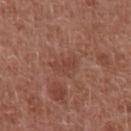The lesion was photographed on a routine skin check and not biopsied; there is no pathology result. Automated image analysis of the tile measured a lesion area of about 4 mm², an eccentricity of roughly 0.85, and a shape-asymmetry score of about 0.35 (0 = symmetric). It also reported a mean CIELAB color near L≈43 a*≈23 b*≈27 and a lesion–skin lightness drop of about 6. The software also gave border irregularity of about 4 on a 0–10 scale. Captured under white-light illumination. A male subject, aged approximately 65. A close-up tile cropped from a whole-body skin photograph, about 15 mm across. Measured at roughly 3 mm in maximum diameter. On the abdomen.